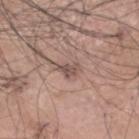Assessment: The lesion was tiled from a total-body skin photograph and was not biopsied. Context: A 15 mm crop from a total-body photograph taken for skin-cancer surveillance. From the head or neck. The patient is a male about 65 years old.